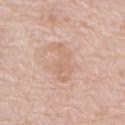follow-up: imaged on a skin check; not biopsied | acquisition: 15 mm crop, total-body photography | patient: female, about 75 years old | body site: the abdomen | tile lighting: white-light.